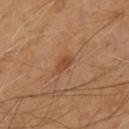Assessment: Part of a total-body skin-imaging series; this lesion was reviewed on a skin check and was not flagged for biopsy. Background: On the front of the torso. Cropped from a whole-body photographic skin survey; the tile spans about 15 mm. Captured under cross-polarized illumination. The recorded lesion diameter is about 2.5 mm. A male patient, aged approximately 55.